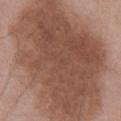Notes:
• follow-up · catalogued during a skin exam; not biopsied
• TBP lesion metrics · a footprint of about 140 mm² and an outline eccentricity of about 0.8 (0 = round, 1 = elongated); an average lesion color of about L≈50 a*≈20 b*≈27 (CIELAB), a lesion–skin lightness drop of about 13, and a lesion-to-skin contrast of about 9.5 (normalized; higher = more distinct); border irregularity of about 3 on a 0–10 scale and peripheral color asymmetry of about 1.5; an automated nevus-likeness rating near 45 out of 100 and a detector confidence of about 100 out of 100 that the crop contains a lesion
• lesion diameter · ~18.5 mm (longest diameter)
• illumination · white-light
• subject · male, about 50 years old
• image source · 15 mm crop, total-body photography
• location · the chest